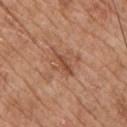Recorded during total-body skin imaging; not selected for excision or biopsy. The tile uses white-light illumination. The lesion is located on the chest. The recorded lesion diameter is about 3.5 mm. The subject is a male aged around 65. A region of skin cropped from a whole-body photographic capture, roughly 15 mm wide.